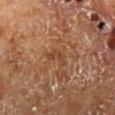This lesion was catalogued during total-body skin photography and was not selected for biopsy.
Automated tile analysis of the lesion measured a shape eccentricity near 0.9 and a shape-asymmetry score of about 0.55 (0 = symmetric). It also reported an average lesion color of about L≈43 a*≈22 b*≈33 (CIELAB), about 6 CIELAB-L* units darker than the surrounding skin, and a normalized border contrast of about 5.5. The analysis additionally found a nevus-likeness score of about 0/100 and a lesion-detection confidence of about 90/100.
Cropped from a total-body skin-imaging series; the visible field is about 15 mm.
The lesion's longest dimension is about 3 mm.
From the right lower leg.
The subject is a male aged 68–72.